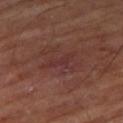Case summary:
• notes · catalogued during a skin exam; not biopsied
• subject · roughly 65 years of age
• anatomic site · the right thigh
• tile lighting · cross-polarized illumination
• acquisition · ~15 mm tile from a whole-body skin photo